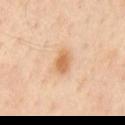{"biopsy_status": "not biopsied; imaged during a skin examination", "patient": {"sex": "male", "age_approx": 55}, "lighting": "cross-polarized", "automated_metrics": {"vs_skin_darker_L": 11.0, "vs_skin_contrast_norm": 8.0, "border_irregularity_0_10": 2.0, "color_variation_0_10": 2.5, "nevus_likeness_0_100": 90, "lesion_detection_confidence_0_100": 100}, "site": "mid back", "image": {"source": "total-body photography crop", "field_of_view_mm": 15}}